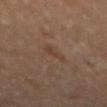follow-up: total-body-photography surveillance lesion; no biopsy
imaging modality: ~15 mm tile from a whole-body skin photo
tile lighting: cross-polarized illumination
site: the back
patient: male, roughly 65 years of age